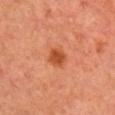Q: Was a biopsy performed?
A: total-body-photography surveillance lesion; no biopsy